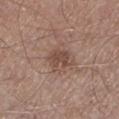Impression: The lesion was tiled from a total-body skin photograph and was not biopsied. Context: A male patient roughly 55 years of age. Cropped from a whole-body photographic skin survey; the tile spans about 15 mm. The lesion is located on the left lower leg. The tile uses white-light illumination.